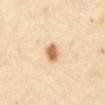lesion_size:
  long_diameter_mm_approx: 2.5
lighting: cross-polarized
image:
  source: total-body photography crop
  field_of_view_mm: 15
patient:
  sex: female
  age_approx: 60
site: abdomen